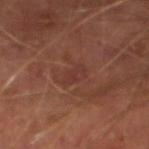Clinical impression:
The lesion was tiled from a total-body skin photograph and was not biopsied.
Context:
The lesion is on the leg. About 2.5 mm across. Cropped from a total-body skin-imaging series; the visible field is about 15 mm. The patient is a male approximately 65 years of age. Imaged with cross-polarized lighting. The total-body-photography lesion software estimated a footprint of about 2.5 mm², an eccentricity of roughly 0.9, and two-axis asymmetry of about 0.35. The software also gave border irregularity of about 4.5 on a 0–10 scale and a within-lesion color-variation index near 0/10.